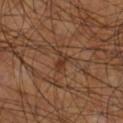This is a cross-polarized tile.
The lesion is on the leg.
The patient is a male aged around 70.
A region of skin cropped from a whole-body photographic capture, roughly 15 mm wide.
Measured at roughly 3 mm in maximum diameter.